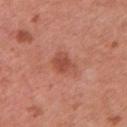notes: imaged on a skin check; not biopsied
lesion size: about 3.5 mm
image: ~15 mm tile from a whole-body skin photo
anatomic site: the left upper arm
patient: female, aged approximately 50